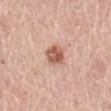follow-up = catalogued during a skin exam; not biopsied.